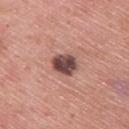workup = total-body-photography surveillance lesion; no biopsy | patient = male, aged 58 to 62 | body site = the upper back | tile lighting = white-light illumination | imaging modality = 15 mm crop, total-body photography.